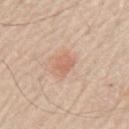<case>
<biopsy_status>not biopsied; imaged during a skin examination</biopsy_status>
<patient>
  <sex>male</sex>
  <age_approx>70</age_approx>
</patient>
<lighting>white-light</lighting>
<site>left upper arm</site>
<image>
  <source>total-body photography crop</source>
  <field_of_view_mm>15</field_of_view_mm>
</image>
</case>